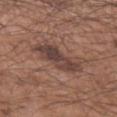<lesion>
<biopsy_status>not biopsied; imaged during a skin examination</biopsy_status>
</lesion>